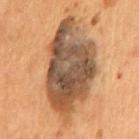<lesion>
  <automated_metrics>
    <cielab_L>47</cielab_L>
    <cielab_a>17</cielab_a>
    <cielab_b>31</cielab_b>
    <vs_skin_darker_L>16.0</vs_skin_darker_L>
    <vs_skin_contrast_norm>12.0</vs_skin_contrast_norm>
    <border_irregularity_0_10>2.5</border_irregularity_0_10>
    <color_variation_0_10>8.5</color_variation_0_10>
    <peripheral_color_asymmetry>2.5</peripheral_color_asymmetry>
  </automated_metrics>
  <site>chest</site>
  <patient>
    <sex>male</sex>
    <age_approx>55</age_approx>
  </patient>
  <image>
    <source>total-body photography crop</source>
    <field_of_view_mm>15</field_of_view_mm>
  </image>
  <lesion_size>
    <long_diameter_mm_approx>12.0</long_diameter_mm_approx>
  </lesion_size>
  <lighting>cross-polarized</lighting>
</lesion>